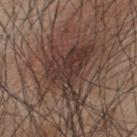Notes:
- notes — catalogued during a skin exam; not biopsied
- illumination — white-light illumination
- anatomic site — the upper back
- imaging modality — ~15 mm crop, total-body skin-cancer survey
- diameter — about 7.5 mm
- patient — male, in their mid- to late 40s